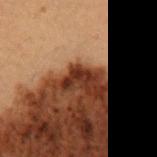About 3 mm across. A male subject aged around 50. Automated image analysis of the tile measured a footprint of about 3.5 mm², a shape eccentricity near 0.9, and a shape-asymmetry score of about 0.65 (0 = symmetric). It also reported a border-irregularity index near 7/10, a within-lesion color-variation index near 0/10, and peripheral color asymmetry of about 0. And it measured a nevus-likeness score of about 5/100 and a lesion-detection confidence of about 65/100. A 15 mm close-up extracted from a 3D total-body photography capture. The lesion is located on the abdomen. Captured under cross-polarized illumination.